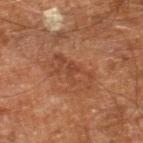Assessment:
Recorded during total-body skin imaging; not selected for excision or biopsy.
Image and clinical context:
The patient is a male aged 58 to 62. Cropped from a total-body skin-imaging series; the visible field is about 15 mm. The lesion is on the right leg. The lesion-visualizer software estimated a lesion area of about 9.5 mm², a shape eccentricity near 0.9, and a symmetry-axis asymmetry near 0.4. And it measured an average lesion color of about L≈43 a*≈23 b*≈32 (CIELAB), about 7 CIELAB-L* units darker than the surrounding skin, and a normalized lesion–skin contrast near 5.5. The software also gave a classifier nevus-likeness of about 5/100 and lesion-presence confidence of about 100/100. This is a cross-polarized tile.